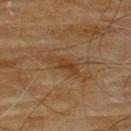  biopsy_status: not biopsied; imaged during a skin examination
  site: chest
  image:
    source: total-body photography crop
    field_of_view_mm: 15
  lesion_size:
    long_diameter_mm_approx: 4.5
  patient:
    sex: male
    age_approx: 60
  lighting: cross-polarized
  automated_metrics:
    area_mm2_approx: 6.0
    shape_asymmetry: 0.35
    border_irregularity_0_10: 4.5
    color_variation_0_10: 2.5
    peripheral_color_asymmetry: 1.0